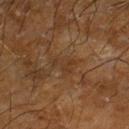| field | value |
|---|---|
| workup | catalogued during a skin exam; not biopsied |
| TBP lesion metrics | lesion-presence confidence of about 70/100 |
| patient | male, about 65 years old |
| size | ~3 mm (longest diameter) |
| lighting | cross-polarized illumination |
| acquisition | 15 mm crop, total-body photography |
| location | the right lower leg |